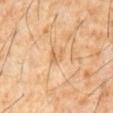This lesion was catalogued during total-body skin photography and was not selected for biopsy.
A male patient, aged approximately 60.
The lesion is on the abdomen.
A roughly 15 mm field-of-view crop from a total-body skin photograph.
About 2.5 mm across.
Imaged with cross-polarized lighting.